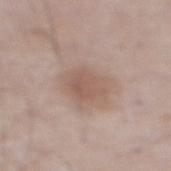follow-up: total-body-photography surveillance lesion; no biopsy
lighting: white-light illumination
location: the abdomen
diameter: ≈3 mm
image: total-body-photography crop, ~15 mm field of view
patient: male, roughly 55 years of age